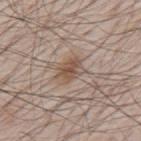{"biopsy_status": "not biopsied; imaged during a skin examination", "image": {"source": "total-body photography crop", "field_of_view_mm": 15}, "lesion_size": {"long_diameter_mm_approx": 3.5}, "site": "mid back", "lighting": "white-light", "patient": {"sex": "male", "age_approx": 80}}